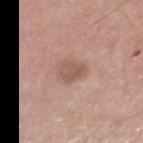Q: What is the anatomic site?
A: the right thigh
Q: Automated lesion metrics?
A: an eccentricity of roughly 0.65 and a shape-asymmetry score of about 0.25 (0 = symmetric); a mean CIELAB color near L≈55 a*≈18 b*≈26 and a lesion–skin lightness drop of about 9; a border-irregularity index near 2.5/10 and radial color variation of about 1; a nevus-likeness score of about 10/100 and a lesion-detection confidence of about 100/100
Q: Patient demographics?
A: male, aged 73–77
Q: What lighting was used for the tile?
A: white-light illumination
Q: What is the lesion's diameter?
A: ≈3 mm
Q: What is the imaging modality?
A: 15 mm crop, total-body photography